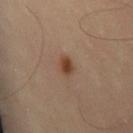Context: The tile uses cross-polarized illumination. A close-up tile cropped from a whole-body skin photograph, about 15 mm across. A male subject aged approximately 55. About 2.5 mm across. The lesion is on the right thigh.